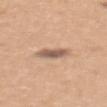Q: Was a biopsy performed?
A: catalogued during a skin exam; not biopsied
Q: Lesion location?
A: the upper back
Q: What kind of image is this?
A: ~15 mm crop, total-body skin-cancer survey
Q: Patient demographics?
A: female, roughly 45 years of age
Q: What is the lesion's diameter?
A: about 3 mm
Q: Automated lesion metrics?
A: a border-irregularity index near 2/10, a color-variation rating of about 4/10, and peripheral color asymmetry of about 1.5; a classifier nevus-likeness of about 40/100 and a detector confidence of about 100 out of 100 that the crop contains a lesion
Q: What lighting was used for the tile?
A: white-light illumination